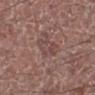biopsy status — catalogued during a skin exam; not biopsied | patient — male, approximately 75 years of age | automated metrics — an average lesion color of about L≈45 a*≈19 b*≈21 (CIELAB), roughly 6 lightness units darker than nearby skin, and a normalized border contrast of about 5; a border-irregularity rating of about 3.5/10, internal color variation of about 3.5 on a 0–10 scale, and a peripheral color-asymmetry measure near 1 | body site — the left lower leg | image source — ~15 mm tile from a whole-body skin photo | illumination — white-light.